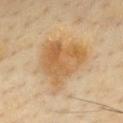  biopsy_status: not biopsied; imaged during a skin examination
  lesion_size:
    long_diameter_mm_approx: 6.5
  image:
    source: total-body photography crop
    field_of_view_mm: 15
  lighting: cross-polarized
  site: chest
  patient:
    sex: male
    age_approx: 55
  automated_metrics:
    cielab_L: 61
    cielab_a: 18
    cielab_b: 41
    vs_skin_darker_L: 10.0
    vs_skin_contrast_norm: 7.5
    nevus_likeness_0_100: 80